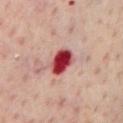The lesion was tiled from a total-body skin photograph and was not biopsied. From the chest. About 3.5 mm across. A region of skin cropped from a whole-body photographic capture, roughly 15 mm wide. A male subject aged around 65. The total-body-photography lesion software estimated a classifier nevus-likeness of about 0/100 and a lesion-detection confidence of about 100/100.